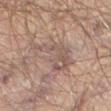The lesion was photographed on a routine skin check and not biopsied; there is no pathology result. Imaged with cross-polarized lighting. The subject is a male roughly 65 years of age. Approximately 5.5 mm at its widest. Located on the right thigh. A 15 mm close-up tile from a total-body photography series done for melanoma screening.